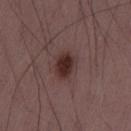| feature | finding |
|---|---|
| workup | imaged on a skin check; not biopsied |
| illumination | white-light illumination |
| image-analysis metrics | a mean CIELAB color near L≈30 a*≈19 b*≈18, a lesion–skin lightness drop of about 11, and a normalized lesion–skin contrast near 10.5; a nevus-likeness score of about 100/100 |
| image source | ~15 mm tile from a whole-body skin photo |
| patient | male, approximately 50 years of age |
| location | the left thigh |